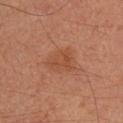This lesion was catalogued during total-body skin photography and was not selected for biopsy. A male patient aged 33 to 37. From the right upper arm. This image is a 15 mm lesion crop taken from a total-body photograph.